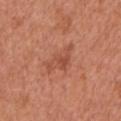Imaged during a routine full-body skin examination; the lesion was not biopsied and no histopathology is available.